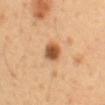• patient · male, approximately 50 years of age
• body site · the abdomen
• imaging modality · 15 mm crop, total-body photography
• TBP lesion metrics · a footprint of about 5.5 mm² and two-axis asymmetry of about 0.2; a classifier nevus-likeness of about 100/100 and a detector confidence of about 100 out of 100 that the crop contains a lesion
• lesion diameter · ≈2.5 mm